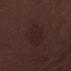Part of a total-body skin-imaging series; this lesion was reviewed on a skin check and was not flagged for biopsy. A male patient in their 50s. The tile uses white-light illumination. Automated image analysis of the tile measured an average lesion color of about L≈21 a*≈17 b*≈16 (CIELAB). It also reported a nevus-likeness score of about 10/100 and a detector confidence of about 100 out of 100 that the crop contains a lesion. A 15 mm close-up extracted from a 3D total-body photography capture. Longest diameter approximately 5 mm.